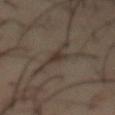Imaged during a routine full-body skin examination; the lesion was not biopsied and no histopathology is available.
A 15 mm close-up extracted from a 3D total-body photography capture.
On the abdomen.
About 2.5 mm across.
The lesion-visualizer software estimated a lesion area of about 3 mm², a shape eccentricity near 0.8, and a symmetry-axis asymmetry near 0.4. The analysis additionally found a mean CIELAB color near L≈32 a*≈10 b*≈20 and a lesion-to-skin contrast of about 7.5 (normalized; higher = more distinct). The software also gave a color-variation rating of about 1.5/10 and peripheral color asymmetry of about 0.5. The software also gave a nevus-likeness score of about 0/100.
Captured under cross-polarized illumination.
A male subject, aged approximately 55.